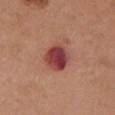The lesion was tiled from a total-body skin photograph and was not biopsied. The lesion is located on the chest. A female patient aged approximately 65. A close-up tile cropped from a whole-body skin photograph, about 15 mm across.